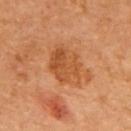Assessment: This lesion was catalogued during total-body skin photography and was not selected for biopsy. Background: The subject is a female aged approximately 65. Located on the upper back. The tile uses cross-polarized illumination. The lesion-visualizer software estimated a shape eccentricity near 0.7 and a symmetry-axis asymmetry near 0.2. The analysis additionally found a lesion–skin lightness drop of about 9 and a normalized border contrast of about 7. The analysis additionally found border irregularity of about 2 on a 0–10 scale, a within-lesion color-variation index near 5.5/10, and radial color variation of about 2. The software also gave a classifier nevus-likeness of about 0/100 and a detector confidence of about 100 out of 100 that the crop contains a lesion. Longest diameter approximately 5.5 mm. Cropped from a whole-body photographic skin survey; the tile spans about 15 mm.